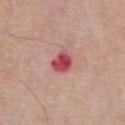Q: Was this lesion biopsied?
A: catalogued during a skin exam; not biopsied
Q: Automated lesion metrics?
A: a shape eccentricity near 0.6; an average lesion color of about L≈50 a*≈36 b*≈22 (CIELAB), about 15 CIELAB-L* units darker than the surrounding skin, and a lesion-to-skin contrast of about 10.5 (normalized; higher = more distinct); a border-irregularity index near 2/10 and a within-lesion color-variation index near 4.5/10
Q: How large is the lesion?
A: ~3 mm (longest diameter)
Q: What are the patient's age and sex?
A: male, aged approximately 75
Q: How was this image acquired?
A: ~15 mm crop, total-body skin-cancer survey
Q: Lesion location?
A: the chest
Q: Illumination type?
A: white-light illumination